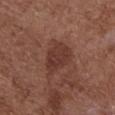A female patient aged around 80.
Imaged with white-light lighting.
The recorded lesion diameter is about 4.5 mm.
This image is a 15 mm lesion crop taken from a total-body photograph.
On the chest.